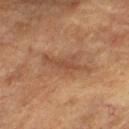notes = catalogued during a skin exam; not biopsied
subject = male, roughly 85 years of age or older
anatomic site = the right lower leg
size = ≈6 mm
image = ~15 mm crop, total-body skin-cancer survey